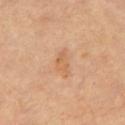Impression:
This lesion was catalogued during total-body skin photography and was not selected for biopsy.
Background:
A 15 mm crop from a total-body photograph taken for skin-cancer surveillance. The patient is aged around 65. Located on the chest. The lesion-visualizer software estimated an average lesion color of about L≈60 a*≈21 b*≈37 (CIELAB), roughly 7 lightness units darker than nearby skin, and a normalized border contrast of about 5.5. The analysis additionally found border irregularity of about 4 on a 0–10 scale, a within-lesion color-variation index near 2.5/10, and radial color variation of about 0.5. And it measured a classifier nevus-likeness of about 0/100 and a lesion-detection confidence of about 100/100. Captured under cross-polarized illumination. Longest diameter approximately 3 mm.